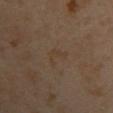notes: catalogued during a skin exam; not biopsied | patient: female, in their 40s | body site: the left upper arm | acquisition: ~15 mm tile from a whole-body skin photo.